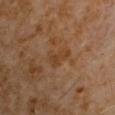| key | value |
|---|---|
| follow-up | no biopsy performed (imaged during a skin exam) |
| image | ~15 mm crop, total-body skin-cancer survey |
| subject | male, aged 58–62 |
| anatomic site | the right upper arm |
| size | ~3.5 mm (longest diameter) |